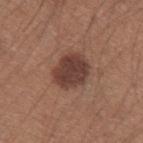{"biopsy_status": "not biopsied; imaged during a skin examination", "image": {"source": "total-body photography crop", "field_of_view_mm": 15}, "lesion_size": {"long_diameter_mm_approx": 4.0}, "site": "left upper arm", "patient": {"sex": "male", "age_approx": 35}, "lighting": "white-light", "automated_metrics": {"border_irregularity_0_10": 1.5}}